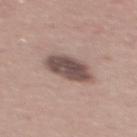Part of a total-body skin-imaging series; this lesion was reviewed on a skin check and was not flagged for biopsy.
The lesion-visualizer software estimated an area of roughly 12 mm², an eccentricity of roughly 0.75, and a shape-asymmetry score of about 0.15 (0 = symmetric). The software also gave a mean CIELAB color near L≈49 a*≈16 b*≈19. The software also gave radial color variation of about 1. The software also gave an automated nevus-likeness rating near 15 out of 100 and lesion-presence confidence of about 100/100.
From the mid back.
A 15 mm close-up tile from a total-body photography series done for melanoma screening.
The patient is a male aged approximately 40.
The tile uses white-light illumination.